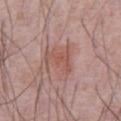No biopsy was performed on this lesion — it was imaged during a full skin examination and was not determined to be concerning. Longest diameter approximately 4.5 mm. A region of skin cropped from a whole-body photographic capture, roughly 15 mm wide. The tile uses white-light illumination. The patient is a male in their mid- to late 60s. The total-body-photography lesion software estimated a lesion-to-skin contrast of about 5.5 (normalized; higher = more distinct). And it measured border irregularity of about 5 on a 0–10 scale, a within-lesion color-variation index near 3.5/10, and a peripheral color-asymmetry measure near 1. On the front of the torso.